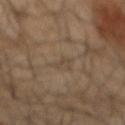Context:
A male subject approximately 65 years of age. The lesion is on the mid back. This is a cross-polarized tile. Cropped from a whole-body photographic skin survey; the tile spans about 15 mm.